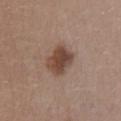A 15 mm crop from a total-body photograph taken for skin-cancer surveillance. Located on the lower back. The patient is a female roughly 35 years of age.